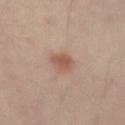From the left upper arm. This image is a 15 mm lesion crop taken from a total-body photograph. Automated tile analysis of the lesion measured a mean CIELAB color near L≈53 a*≈19 b*≈27 and a lesion-to-skin contrast of about 7 (normalized; higher = more distinct). And it measured a border-irregularity rating of about 2.5/10, internal color variation of about 2.5 on a 0–10 scale, and a peripheral color-asymmetry measure near 1. The software also gave an automated nevus-likeness rating near 95 out of 100 and lesion-presence confidence of about 100/100. A subject in their mid-50s. Longest diameter approximately 3 mm.